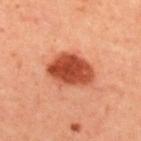Findings:
• biopsy status: total-body-photography surveillance lesion; no biopsy
• subject: female, roughly 45 years of age
• location: the upper back
• acquisition: ~15 mm tile from a whole-body skin photo
• size: about 5.5 mm
• tile lighting: cross-polarized illumination